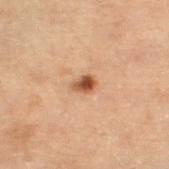notes: no biopsy performed (imaged during a skin exam) | imaging modality: ~15 mm tile from a whole-body skin photo | subject: male, approximately 70 years of age | lighting: cross-polarized | image-analysis metrics: a border-irregularity rating of about 2/10 and peripheral color asymmetry of about 2.5; a nevus-likeness score of about 95/100 and a detector confidence of about 100 out of 100 that the crop contains a lesion | size: ≈2.5 mm | location: the right thigh.